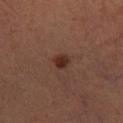Findings:
- site: the left lower leg
- patient: male, aged 33–37
- imaging modality: ~15 mm crop, total-body skin-cancer survey
- automated metrics: a lesion area of about 3.5 mm², a shape eccentricity near 0.5, and a shape-asymmetry score of about 0.2 (0 = symmetric); an average lesion color of about L≈28 a*≈19 b*≈23 (CIELAB), about 9 CIELAB-L* units darker than the surrounding skin, and a normalized lesion–skin contrast near 9.5; border irregularity of about 1.5 on a 0–10 scale, internal color variation of about 3 on a 0–10 scale, and radial color variation of about 1; a nevus-likeness score of about 95/100 and a lesion-detection confidence of about 100/100